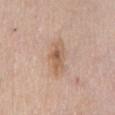Clinical impression:
Imaged during a routine full-body skin examination; the lesion was not biopsied and no histopathology is available.
Background:
On the chest. Automated image analysis of the tile measured a lesion color around L≈60 a*≈18 b*≈31 in CIELAB, roughly 9 lightness units darker than nearby skin, and a normalized lesion–skin contrast near 7. The analysis additionally found an automated nevus-likeness rating near 5 out of 100 and a detector confidence of about 100 out of 100 that the crop contains a lesion. A roughly 15 mm field-of-view crop from a total-body skin photograph. A female subject aged approximately 65.